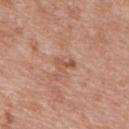Recorded during total-body skin imaging; not selected for excision or biopsy.
Located on the mid back.
The tile uses white-light illumination.
A male patient about 50 years old.
Approximately 2.5 mm at its widest.
A 15 mm close-up extracted from a 3D total-body photography capture.